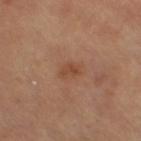Q: Automated lesion metrics?
A: border irregularity of about 2 on a 0–10 scale and a color-variation rating of about 2.5/10; an automated nevus-likeness rating near 15 out of 100 and a detector confidence of about 100 out of 100 that the crop contains a lesion
Q: Lesion size?
A: ≈2.5 mm
Q: Who is the patient?
A: female, aged 58–62
Q: Lesion location?
A: the left leg
Q: Illumination type?
A: cross-polarized
Q: How was this image acquired?
A: total-body-photography crop, ~15 mm field of view